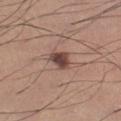The lesion was photographed on a routine skin check and not biopsied; there is no pathology result. A close-up tile cropped from a whole-body skin photograph, about 15 mm across. The total-body-photography lesion software estimated a border-irregularity index near 2.5/10 and a color-variation rating of about 3/10. The software also gave an automated nevus-likeness rating near 85 out of 100 and a lesion-detection confidence of about 100/100. Approximately 3 mm at its widest. Located on the left lower leg. The patient is a male aged 58 to 62. Imaged with white-light lighting.